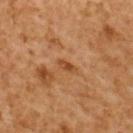Impression:
Captured during whole-body skin photography for melanoma surveillance; the lesion was not biopsied.
Background:
The patient is a male approximately 60 years of age. The lesion is on the upper back. The recorded lesion diameter is about 2.5 mm. A region of skin cropped from a whole-body photographic capture, roughly 15 mm wide. Imaged with cross-polarized lighting.